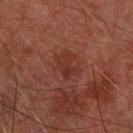{"biopsy_status": "not biopsied; imaged during a skin examination", "site": "right forearm", "image": {"source": "total-body photography crop", "field_of_view_mm": 15}, "patient": {"sex": "male", "age_approx": 75}}